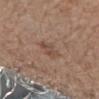Recorded during total-body skin imaging; not selected for excision or biopsy. This is a white-light tile. From the right forearm. The lesion's longest dimension is about 3 mm. The patient is a male aged around 70. A lesion tile, about 15 mm wide, cut from a 3D total-body photograph.